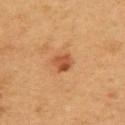Impression:
No biopsy was performed on this lesion — it was imaged during a full skin examination and was not determined to be concerning.
Acquisition and patient details:
The lesion is located on the upper back. The patient is a male aged 53–57. This is a cross-polarized tile. Approximately 2.5 mm at its widest. Automated tile analysis of the lesion measured a mean CIELAB color near L≈46 a*≈24 b*≈35 and roughly 10 lightness units darker than nearby skin. A lesion tile, about 15 mm wide, cut from a 3D total-body photograph.